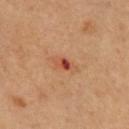Imaged during a routine full-body skin examination; the lesion was not biopsied and no histopathology is available. This image is a 15 mm lesion crop taken from a total-body photograph. The patient is a male roughly 50 years of age. Automated tile analysis of the lesion measured a border-irregularity index near 3.5/10, internal color variation of about 9.5 on a 0–10 scale, and radial color variation of about 3. And it measured a nevus-likeness score of about 0/100 and lesion-presence confidence of about 100/100. From the chest.